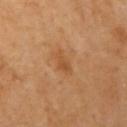Q: Was a biopsy performed?
A: catalogued during a skin exam; not biopsied
Q: What are the patient's age and sex?
A: female, roughly 70 years of age
Q: What is the lesion's diameter?
A: ~3 mm (longest diameter)
Q: How was the tile lit?
A: cross-polarized
Q: How was this image acquired?
A: ~15 mm crop, total-body skin-cancer survey
Q: Lesion location?
A: the right upper arm